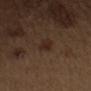The lesion was photographed on a routine skin check and not biopsied; there is no pathology result. A male subject, about 70 years old. A region of skin cropped from a whole-body photographic capture, roughly 15 mm wide. Measured at roughly 2.5 mm in maximum diameter. The lesion is located on the right upper arm.